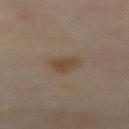This lesion was catalogued during total-body skin photography and was not selected for biopsy. The lesion is located on the abdomen. Cropped from a total-body skin-imaging series; the visible field is about 15 mm. A female patient, aged approximately 50.